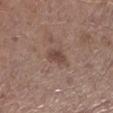– biopsy status · catalogued during a skin exam; not biopsied
– illumination · white-light
– anatomic site · the left lower leg
– lesion size · ≈2.5 mm
– subject · male, about 60 years old
– image source · 15 mm crop, total-body photography